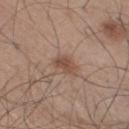{
  "biopsy_status": "not biopsied; imaged during a skin examination",
  "patient": {
    "sex": "male",
    "age_approx": 45
  },
  "image": {
    "source": "total-body photography crop",
    "field_of_view_mm": 15
  },
  "lesion_size": {
    "long_diameter_mm_approx": 3.0
  },
  "lighting": "white-light",
  "site": "right thigh"
}